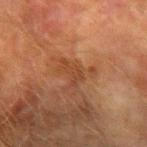{"biopsy_status": "not biopsied; imaged during a skin examination", "lesion_size": {"long_diameter_mm_approx": 3.0}, "lighting": "cross-polarized", "patient": {"sex": "male", "age_approx": 75}, "image": {"source": "total-body photography crop", "field_of_view_mm": 15}, "site": "right forearm"}